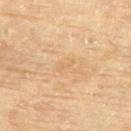A 15 mm close-up extracted from a 3D total-body photography capture.
Longest diameter approximately 2 mm.
A female subject aged 78–82.
From the upper back.
Captured under cross-polarized illumination.